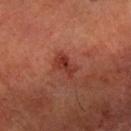Impression: This lesion was catalogued during total-body skin photography and was not selected for biopsy. Clinical summary: A close-up tile cropped from a whole-body skin photograph, about 15 mm across. The total-body-photography lesion software estimated a footprint of about 5 mm² and an eccentricity of roughly 0.8. And it measured a mean CIELAB color near L≈34 a*≈28 b*≈28, roughly 8 lightness units darker than nearby skin, and a normalized border contrast of about 7.5. On the right forearm. The tile uses cross-polarized illumination. Longest diameter approximately 3 mm. A male subject aged around 60.